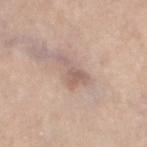notes = no biopsy performed (imaged during a skin exam)
imaging modality = ~15 mm crop, total-body skin-cancer survey
patient = female, aged around 65
site = the left thigh
tile lighting = white-light
diameter = ~4.5 mm (longest diameter)